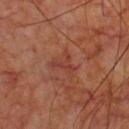Part of a total-body skin-imaging series; this lesion was reviewed on a skin check and was not flagged for biopsy. A 15 mm close-up tile from a total-body photography series done for melanoma screening. Located on the chest. This is a cross-polarized tile. The lesion's longest dimension is about 2.5 mm. A male patient aged around 65. Automated image analysis of the tile measured border irregularity of about 7.5 on a 0–10 scale and radial color variation of about 0. And it measured a nevus-likeness score of about 0/100 and a detector confidence of about 100 out of 100 that the crop contains a lesion.